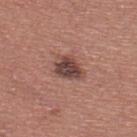Q: Is there a histopathology result?
A: imaged on a skin check; not biopsied
Q: Patient demographics?
A: male, roughly 40 years of age
Q: Illumination type?
A: white-light
Q: What did automated image analysis measure?
A: a lesion area of about 7 mm² and an outline eccentricity of about 0.65 (0 = round, 1 = elongated); a mean CIELAB color near L≈43 a*≈21 b*≈22, a lesion–skin lightness drop of about 14, and a normalized lesion–skin contrast near 10.5; border irregularity of about 2 on a 0–10 scale, a within-lesion color-variation index near 4.5/10, and peripheral color asymmetry of about 1.5; an automated nevus-likeness rating near 25 out of 100 and lesion-presence confidence of about 100/100
Q: What is the anatomic site?
A: the upper back
Q: What is the lesion's diameter?
A: ≈3.5 mm
Q: How was this image acquired?
A: ~15 mm crop, total-body skin-cancer survey